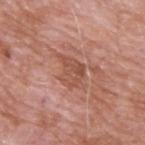workup=no biopsy performed (imaged during a skin exam)
site=the back
imaging modality=~15 mm tile from a whole-body skin photo
patient=male, approximately 60 years of age